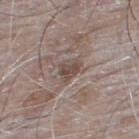body site: the upper back
TBP lesion metrics: an average lesion color of about L≈47 a*≈14 b*≈21 (CIELAB), about 9 CIELAB-L* units darker than the surrounding skin, and a normalized lesion–skin contrast near 7; a border-irregularity rating of about 3.5/10; a classifier nevus-likeness of about 0/100 and a lesion-detection confidence of about 55/100
tile lighting: white-light illumination
acquisition: 15 mm crop, total-body photography
patient: male, aged 63–67